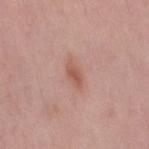Q: What lighting was used for the tile?
A: white-light illumination
Q: Automated lesion metrics?
A: an average lesion color of about L≈56 a*≈23 b*≈28 (CIELAB), a lesion–skin lightness drop of about 9, and a lesion-to-skin contrast of about 7 (normalized; higher = more distinct); a border-irregularity rating of about 2.5/10 and a within-lesion color-variation index near 2/10
Q: What kind of image is this?
A: total-body-photography crop, ~15 mm field of view
Q: What is the lesion's diameter?
A: about 3 mm
Q: Lesion location?
A: the right thigh
Q: Patient demographics?
A: female, approximately 50 years of age